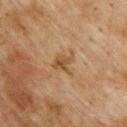On the upper back.
The patient is a male aged 73 to 77.
This image is a 15 mm lesion crop taken from a total-body photograph.